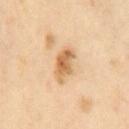<lesion>
  <biopsy_status>not biopsied; imaged during a skin examination</biopsy_status>
  <image>
    <source>total-body photography crop</source>
    <field_of_view_mm>15</field_of_view_mm>
  </image>
  <lighting>cross-polarized</lighting>
  <automated_metrics>
    <nevus_likeness_0_100>30</nevus_likeness_0_100>
  </automated_metrics>
  <site>chest</site>
  <lesion_size>
    <long_diameter_mm_approx>4.0</long_diameter_mm_approx>
  </lesion_size>
  <patient>
    <sex>female</sex>
    <age_approx>55</age_approx>
  </patient>
</lesion>